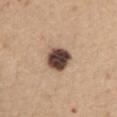Impression:
The lesion was tiled from a total-body skin photograph and was not biopsied.
Acquisition and patient details:
A female subject in their mid-40s. A region of skin cropped from a whole-body photographic capture, roughly 15 mm wide. Longest diameter approximately 3.5 mm. The tile uses white-light illumination. On the front of the torso. An algorithmic analysis of the crop reported an area of roughly 10 mm² and two-axis asymmetry of about 0.15. The software also gave a classifier nevus-likeness of about 60/100 and a lesion-detection confidence of about 100/100.